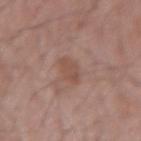Q: Was a biopsy performed?
A: imaged on a skin check; not biopsied
Q: What is the imaging modality?
A: ~15 mm tile from a whole-body skin photo
Q: How large is the lesion?
A: about 2.5 mm
Q: Illumination type?
A: white-light
Q: Where on the body is the lesion?
A: the arm
Q: Patient demographics?
A: male, about 55 years old